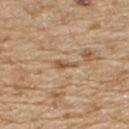<lesion>
  <biopsy_status>not biopsied; imaged during a skin examination</biopsy_status>
  <image>
    <source>total-body photography crop</source>
    <field_of_view_mm>15</field_of_view_mm>
  </image>
  <lighting>white-light</lighting>
  <automated_metrics>
    <nevus_likeness_0_100>0</nevus_likeness_0_100>
    <lesion_detection_confidence_0_100>65</lesion_detection_confidence_0_100>
  </automated_metrics>
  <site>back</site>
  <lesion_size>
    <long_diameter_mm_approx>3.0</long_diameter_mm_approx>
  </lesion_size>
  <patient>
    <sex>male</sex>
    <age_approx>70</age_approx>
  </patient>
</lesion>